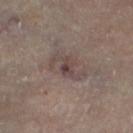workup = total-body-photography surveillance lesion; no biopsy | illumination = cross-polarized | patient = female, in their 60s | image source = total-body-photography crop, ~15 mm field of view | size = ≈4 mm | image-analysis metrics = a footprint of about 6 mm², an eccentricity of roughly 0.85, and a symmetry-axis asymmetry near 0.5; a mean CIELAB color near L≈42 a*≈14 b*≈17, a lesion–skin lightness drop of about 8, and a normalized border contrast of about 7; a border-irregularity rating of about 8/10, internal color variation of about 6 on a 0–10 scale, and a peripheral color-asymmetry measure near 2 | site = the left thigh.